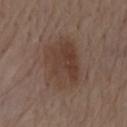Part of a total-body skin-imaging series; this lesion was reviewed on a skin check and was not flagged for biopsy. Captured under white-light illumination. A lesion tile, about 15 mm wide, cut from a 3D total-body photograph. The lesion is on the mid back. Longest diameter approximately 6.5 mm. An algorithmic analysis of the crop reported a lesion area of about 22 mm², an outline eccentricity of about 0.75 (0 = round, 1 = elongated), and a shape-asymmetry score of about 0.15 (0 = symmetric). The analysis additionally found a nevus-likeness score of about 70/100 and a lesion-detection confidence of about 100/100. The subject is a male approximately 70 years of age.